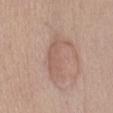{"biopsy_status": "not biopsied; imaged during a skin examination", "lesion_size": {"long_diameter_mm_approx": 5.5}, "site": "abdomen", "automated_metrics": {"eccentricity": 0.9, "shape_asymmetry": 0.4}, "lighting": "white-light", "image": {"source": "total-body photography crop", "field_of_view_mm": 15}, "patient": {"sex": "female", "age_approx": 55}}